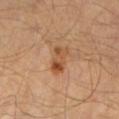The lesion was tiled from a total-body skin photograph and was not biopsied. A 15 mm crop from a total-body photograph taken for skin-cancer surveillance. About 3.5 mm across. An algorithmic analysis of the crop reported a symmetry-axis asymmetry near 0.25. From the left lower leg. The tile uses cross-polarized illumination. The patient is a male aged 38 to 42.